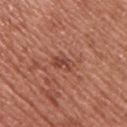The lesion was tiled from a total-body skin photograph and was not biopsied. A 15 mm close-up tile from a total-body photography series done for melanoma screening. The patient is a male in their mid-50s. The lesion is located on the upper back.